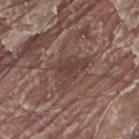Case summary:
- biopsy status: total-body-photography surveillance lesion; no biopsy
- imaging modality: 15 mm crop, total-body photography
- anatomic site: the upper back
- subject: male, roughly 40 years of age
- tile lighting: white-light
- diameter: about 5 mm